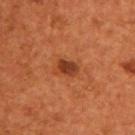biopsy status: no biopsy performed (imaged during a skin exam) | subject: male, approximately 55 years of age | location: the upper back | tile lighting: cross-polarized illumination | image: ~15 mm tile from a whole-body skin photo.